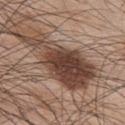Assessment: Recorded during total-body skin imaging; not selected for excision or biopsy. Context: The tile uses white-light illumination. From the upper back. The patient is a male in their mid- to late 40s. Longest diameter approximately 10 mm. Cropped from a whole-body photographic skin survey; the tile spans about 15 mm.